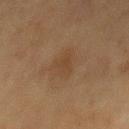Clinical impression:
The lesion was photographed on a routine skin check and not biopsied; there is no pathology result.
Context:
This image is a 15 mm lesion crop taken from a total-body photograph. Captured under cross-polarized illumination. An algorithmic analysis of the crop reported a lesion area of about 6.5 mm² and an eccentricity of roughly 0.85. The analysis additionally found a border-irregularity index near 4.5/10, a color-variation rating of about 1.5/10, and peripheral color asymmetry of about 0.5. The lesion is located on the mid back. The subject is a male about 75 years old.